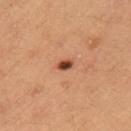{
  "biopsy_status": "not biopsied; imaged during a skin examination",
  "automated_metrics": {
    "border_irregularity_0_10": 2.0,
    "color_variation_0_10": 3.0,
    "peripheral_color_asymmetry": 1.0,
    "nevus_likeness_0_100": 100,
    "lesion_detection_confidence_0_100": 100
  },
  "patient": {
    "sex": "female",
    "age_approx": 35
  },
  "image": {
    "source": "total-body photography crop",
    "field_of_view_mm": 15
  },
  "lighting": "cross-polarized",
  "lesion_size": {
    "long_diameter_mm_approx": 2.0
  },
  "site": "left upper arm"
}